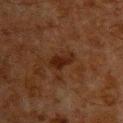notes: total-body-photography surveillance lesion; no biopsy
size: ≈4 mm
patient: male, aged approximately 60
image: ~15 mm crop, total-body skin-cancer survey
site: the upper back
TBP lesion metrics: about 7 CIELAB-L* units darker than the surrounding skin; a border-irregularity rating of about 4/10, a color-variation rating of about 1/10, and peripheral color asymmetry of about 0.5